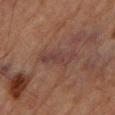Q: Was this lesion biopsied?
A: catalogued during a skin exam; not biopsied
Q: How was this image acquired?
A: ~15 mm crop, total-body skin-cancer survey
Q: How large is the lesion?
A: ≈5 mm
Q: What are the patient's age and sex?
A: male, in their mid-80s or older
Q: Lesion location?
A: the left lower leg